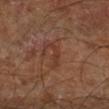Q: Was this lesion biopsied?
A: total-body-photography surveillance lesion; no biopsy
Q: How was this image acquired?
A: total-body-photography crop, ~15 mm field of view
Q: What did automated image analysis measure?
A: a mean CIELAB color near L≈35 a*≈22 b*≈27, a lesion–skin lightness drop of about 6, and a lesion-to-skin contrast of about 5.5 (normalized; higher = more distinct); a border-irregularity index near 9/10 and a color-variation rating of about 0/10; a detector confidence of about 100 out of 100 that the crop contains a lesion
Q: How was the tile lit?
A: cross-polarized illumination
Q: What are the patient's age and sex?
A: aged approximately 65
Q: What is the anatomic site?
A: the right lower leg
Q: Lesion size?
A: about 4 mm